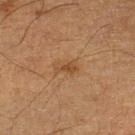Image and clinical context: Approximately 3 mm at its widest. On the left lower leg. This is a cross-polarized tile. A 15 mm close-up extracted from a 3D total-body photography capture. The subject is a male aged around 75. The total-body-photography lesion software estimated about 6 CIELAB-L* units darker than the surrounding skin and a lesion-to-skin contrast of about 6 (normalized; higher = more distinct). And it measured a border-irregularity index near 4/10, internal color variation of about 1 on a 0–10 scale, and a peripheral color-asymmetry measure near 0.5.